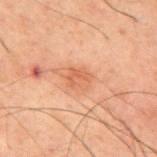No biopsy was performed on this lesion — it was imaged during a full skin examination and was not determined to be concerning.
A male subject, in their 60s.
The lesion is located on the upper back.
A region of skin cropped from a whole-body photographic capture, roughly 15 mm wide.
Imaged with cross-polarized lighting.
The lesion's longest dimension is about 2.5 mm.
The total-body-photography lesion software estimated a border-irregularity index near 2/10, a color-variation rating of about 3/10, and a peripheral color-asymmetry measure near 1. It also reported a nevus-likeness score of about 5/100 and a detector confidence of about 100 out of 100 that the crop contains a lesion.